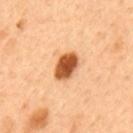Assessment:
Part of a total-body skin-imaging series; this lesion was reviewed on a skin check and was not flagged for biopsy.
Acquisition and patient details:
The subject is a male aged around 50. An algorithmic analysis of the crop reported a nevus-likeness score of about 100/100 and lesion-presence confidence of about 100/100. Imaged with cross-polarized lighting. Cropped from a whole-body photographic skin survey; the tile spans about 15 mm. On the mid back. Approximately 4 mm at its widest.